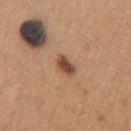{
  "biopsy_status": "not biopsied; imaged during a skin examination",
  "lighting": "white-light",
  "image": {
    "source": "total-body photography crop",
    "field_of_view_mm": 15
  },
  "patient": {
    "sex": "male",
    "age_approx": 65
  },
  "site": "chest",
  "lesion_size": {
    "long_diameter_mm_approx": 2.5
  }
}